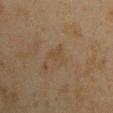Part of a total-body skin-imaging series; this lesion was reviewed on a skin check and was not flagged for biopsy.
From the left upper arm.
This is a cross-polarized tile.
The subject is a male aged 43–47.
A roughly 15 mm field-of-view crop from a total-body skin photograph.
Measured at roughly 2.5 mm in maximum diameter.